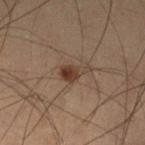Notes:
– biopsy status — imaged on a skin check; not biopsied
– subject — male, aged 53–57
– imaging modality — ~15 mm tile from a whole-body skin photo
– tile lighting — cross-polarized illumination
– anatomic site — the left lower leg
– lesion size — about 3.5 mm